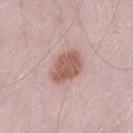Recorded during total-body skin imaging; not selected for excision or biopsy. A male patient, aged 48 to 52. Located on the left thigh. A 15 mm close-up extracted from a 3D total-body photography capture.